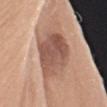This lesion was catalogued during total-body skin photography and was not selected for biopsy.
The lesion is on the left upper arm.
A 15 mm crop from a total-body photograph taken for skin-cancer surveillance.
A female subject, aged around 60.
Automated image analysis of the tile measured roughly 13 lightness units darker than nearby skin and a normalized border contrast of about 9. It also reported border irregularity of about 2 on a 0–10 scale and a color-variation rating of about 5.5/10.
Approximately 6.5 mm at its widest.
This is a white-light tile.